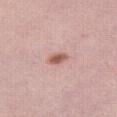No biopsy was performed on this lesion — it was imaged during a full skin examination and was not determined to be concerning. Longest diameter approximately 2.5 mm. Captured under white-light illumination. Automated image analysis of the tile measured an average lesion color of about L≈58 a*≈22 b*≈25 (CIELAB) and a normalized border contrast of about 8.5. The software also gave a nevus-likeness score of about 90/100. From the right thigh. A female subject aged around 55. This image is a 15 mm lesion crop taken from a total-body photograph.